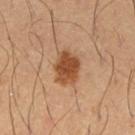This lesion was catalogued during total-body skin photography and was not selected for biopsy. A male patient in their 40s. The lesion is on the arm. A roughly 15 mm field-of-view crop from a total-body skin photograph.